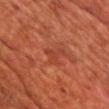Impression:
Imaged during a routine full-body skin examination; the lesion was not biopsied and no histopathology is available.
Background:
Captured under cross-polarized illumination. The subject is a male about 70 years old. Cropped from a total-body skin-imaging series; the visible field is about 15 mm. On the chest. Measured at roughly 3 mm in maximum diameter.